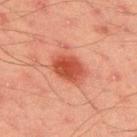Impression: Recorded during total-body skin imaging; not selected for excision or biopsy.